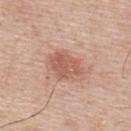Q: Was a biopsy performed?
A: total-body-photography surveillance lesion; no biopsy
Q: Who is the patient?
A: male, aged approximately 55
Q: How was this image acquired?
A: 15 mm crop, total-body photography
Q: Where on the body is the lesion?
A: the upper back
Q: How was the tile lit?
A: white-light illumination
Q: Automated lesion metrics?
A: a lesion color around L≈58 a*≈23 b*≈29 in CIELAB, a lesion–skin lightness drop of about 10, and a normalized lesion–skin contrast near 7; border irregularity of about 2.5 on a 0–10 scale; a lesion-detection confidence of about 100/100
Q: How large is the lesion?
A: about 4 mm